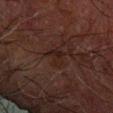Impression: The lesion was tiled from a total-body skin photograph and was not biopsied. Context: A male patient in their 70s. The lesion is on the left forearm. Cropped from a whole-body photographic skin survey; the tile spans about 15 mm. The lesion-visualizer software estimated a lesion–skin lightness drop of about 4 and a lesion-to-skin contrast of about 6 (normalized; higher = more distinct). The analysis additionally found border irregularity of about 4.5 on a 0–10 scale, a color-variation rating of about 2.5/10, and radial color variation of about 1.